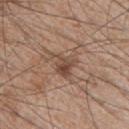workup: no biopsy performed (imaged during a skin exam)
patient: male, aged 48–52
site: the chest
image source: total-body-photography crop, ~15 mm field of view
automated metrics: a footprint of about 6.5 mm² and a shape-asymmetry score of about 0.5 (0 = symmetric); a peripheral color-asymmetry measure near 2.5
illumination: white-light illumination
diameter: about 3.5 mm